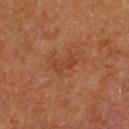biopsy_status: not biopsied; imaged during a skin examination
site: right forearm
patient:
  sex: female
  age_approx: 55
automated_metrics:
  area_mm2_approx: 3.0
  eccentricity: 0.9
  shape_asymmetry: 0.4
  color_variation_0_10: 0.0
  peripheral_color_asymmetry: 0.0
image:
  source: total-body photography crop
  field_of_view_mm: 15
lesion_size:
  long_diameter_mm_approx: 3.0
lighting: cross-polarized